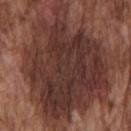Part of a total-body skin-imaging series; this lesion was reviewed on a skin check and was not flagged for biopsy. A male patient aged 73 to 77. A region of skin cropped from a whole-body photographic capture, roughly 15 mm wide. About 15 mm across. The lesion is on the chest. An algorithmic analysis of the crop reported an area of roughly 85 mm², an eccentricity of roughly 0.7, and a symmetry-axis asymmetry near 0.3. The software also gave a mean CIELAB color near L≈34 a*≈20 b*≈22, about 12 CIELAB-L* units darker than the surrounding skin, and a normalized lesion–skin contrast near 11. The analysis additionally found border irregularity of about 5.5 on a 0–10 scale and internal color variation of about 5 on a 0–10 scale.